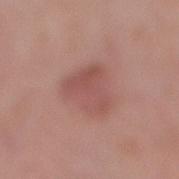Q: Was a biopsy performed?
A: catalogued during a skin exam; not biopsied
Q: Lesion location?
A: the right lower leg
Q: Lesion size?
A: ≈5 mm
Q: Patient demographics?
A: female, aged 38 to 42
Q: What lighting was used for the tile?
A: white-light
Q: What did automated image analysis measure?
A: an automated nevus-likeness rating near 55 out of 100 and a detector confidence of about 100 out of 100 that the crop contains a lesion
Q: What is the imaging modality?
A: ~15 mm crop, total-body skin-cancer survey